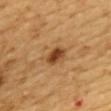The lesion-visualizer software estimated a lesion area of about 4.5 mm², an outline eccentricity of about 0.7 (0 = round, 1 = elongated), and two-axis asymmetry of about 0.2. And it measured a classifier nevus-likeness of about 95/100 and a lesion-detection confidence of about 100/100. A close-up tile cropped from a whole-body skin photograph, about 15 mm across. This is a cross-polarized tile. The recorded lesion diameter is about 3 mm. The subject is a female aged around 55. Located on the upper back.